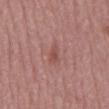Q: Was a biopsy performed?
A: imaged on a skin check; not biopsied
Q: How large is the lesion?
A: ≈3 mm
Q: What kind of image is this?
A: 15 mm crop, total-body photography
Q: Where on the body is the lesion?
A: the mid back
Q: How was the tile lit?
A: white-light illumination
Q: What are the patient's age and sex?
A: male, in their 50s